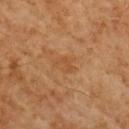Case summary:
- notes — total-body-photography surveillance lesion; no biopsy
- lighting — cross-polarized illumination
- subject — male, aged 58–62
- body site — the mid back
- acquisition — 15 mm crop, total-body photography
- TBP lesion metrics — a lesion area of about 3 mm² and an eccentricity of roughly 0.8